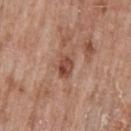Q: Was a biopsy performed?
A: catalogued during a skin exam; not biopsied
Q: Who is the patient?
A: male, aged approximately 65
Q: Automated lesion metrics?
A: an eccentricity of roughly 0.7 and a symmetry-axis asymmetry near 0.2; a mean CIELAB color near L≈48 a*≈23 b*≈29, about 11 CIELAB-L* units darker than the surrounding skin, and a normalized lesion–skin contrast near 8; border irregularity of about 2 on a 0–10 scale, internal color variation of about 5.5 on a 0–10 scale, and a peripheral color-asymmetry measure near 2; a nevus-likeness score of about 0/100 and a detector confidence of about 100 out of 100 that the crop contains a lesion
Q: How was this image acquired?
A: total-body-photography crop, ~15 mm field of view
Q: What is the anatomic site?
A: the mid back
Q: What is the lesion's diameter?
A: ~3 mm (longest diameter)